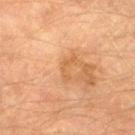Notes:
- workup · no biopsy performed (imaged during a skin exam)
- anatomic site · the right thigh
- subject · male, about 75 years old
- lighting · cross-polarized illumination
- acquisition · 15 mm crop, total-body photography